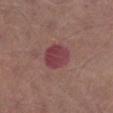Clinical impression: Imaged during a routine full-body skin examination; the lesion was not biopsied and no histopathology is available.